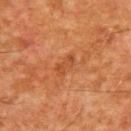The lesion was photographed on a routine skin check and not biopsied; there is no pathology result. On the upper back. The subject is a male aged 63–67. Automated tile analysis of the lesion measured a mean CIELAB color near L≈39 a*≈24 b*≈34, roughly 6 lightness units darker than nearby skin, and a lesion-to-skin contrast of about 5.5 (normalized; higher = more distinct). The software also gave a nevus-likeness score of about 0/100 and a detector confidence of about 100 out of 100 that the crop contains a lesion. Imaged with cross-polarized lighting. Cropped from a total-body skin-imaging series; the visible field is about 15 mm.